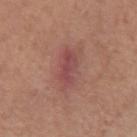The lesion-visualizer software estimated border irregularity of about 3 on a 0–10 scale, internal color variation of about 4.5 on a 0–10 scale, and a peripheral color-asymmetry measure near 1.5.
Captured under white-light illumination.
The recorded lesion diameter is about 4 mm.
Located on the right forearm.
A 15 mm crop from a total-body photograph taken for skin-cancer surveillance.
The subject is a female in their mid-60s.